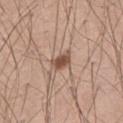Q: Was this lesion biopsied?
A: catalogued during a skin exam; not biopsied
Q: Lesion location?
A: the left thigh
Q: How was this image acquired?
A: ~15 mm tile from a whole-body skin photo
Q: What lighting was used for the tile?
A: white-light illumination
Q: Lesion size?
A: about 3 mm
Q: Who is the patient?
A: male, roughly 40 years of age